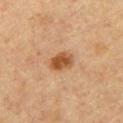Clinical impression: No biopsy was performed on this lesion — it was imaged during a full skin examination and was not determined to be concerning. Image and clinical context: A male patient roughly 60 years of age. A 15 mm crop from a total-body photograph taken for skin-cancer surveillance.